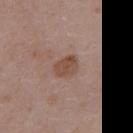biopsy status: total-body-photography surveillance lesion; no biopsy
site: the front of the torso
image-analysis metrics: an outline eccentricity of about 0.6 (0 = round, 1 = elongated) and a symmetry-axis asymmetry near 0.2; an average lesion color of about L≈48 a*≈19 b*≈26 (CIELAB)
patient: female, approximately 35 years of age
lesion size: about 3 mm
image source: 15 mm crop, total-body photography
tile lighting: white-light